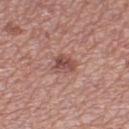follow-up: imaged on a skin check; not biopsied.